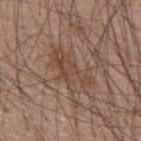This lesion was catalogued during total-body skin photography and was not selected for biopsy.
The lesion is located on the upper back.
The tile uses white-light illumination.
A male subject about 60 years old.
Cropped from a total-body skin-imaging series; the visible field is about 15 mm.
Longest diameter approximately 6.5 mm.
An algorithmic analysis of the crop reported an area of roughly 15 mm², an eccentricity of roughly 0.85, and two-axis asymmetry of about 0.35. The analysis additionally found an automated nevus-likeness rating near 0 out of 100 and lesion-presence confidence of about 70/100.